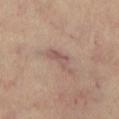Impression: Recorded during total-body skin imaging; not selected for excision or biopsy. Clinical summary: The subject is a female aged 63 to 67. About 4 mm across. Captured under cross-polarized illumination. The lesion is located on the leg. A roughly 15 mm field-of-view crop from a total-body skin photograph.